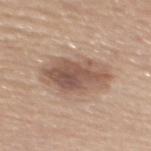Imaged during a routine full-body skin examination; the lesion was not biopsied and no histopathology is available.